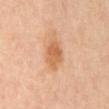Assessment:
Captured during whole-body skin photography for melanoma surveillance; the lesion was not biopsied.
Background:
About 4 mm across. The lesion-visualizer software estimated roughly 10 lightness units darker than nearby skin and a normalized border contrast of about 7. And it measured a border-irregularity index near 2/10 and radial color variation of about 1.5. The lesion is located on the mid back. A lesion tile, about 15 mm wide, cut from a 3D total-body photograph. This is a cross-polarized tile.